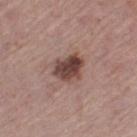Notes:
• notes — catalogued during a skin exam; not biopsied
• anatomic site — the left thigh
• acquisition — 15 mm crop, total-body photography
• lesion diameter — ≈3.5 mm
• patient — female, approximately 65 years of age